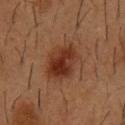This lesion was catalogued during total-body skin photography and was not selected for biopsy.
The tile uses cross-polarized illumination.
A 15 mm close-up tile from a total-body photography series done for melanoma screening.
A male subject aged 53–57.
About 5 mm across.
Automated tile analysis of the lesion measured a lesion color around L≈29 a*≈20 b*≈27 in CIELAB, about 9 CIELAB-L* units darker than the surrounding skin, and a lesion-to-skin contrast of about 9 (normalized; higher = more distinct). The analysis additionally found a within-lesion color-variation index near 5.5/10 and peripheral color asymmetry of about 2. And it measured an automated nevus-likeness rating near 100 out of 100 and a detector confidence of about 100 out of 100 that the crop contains a lesion.
Located on the chest.